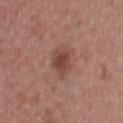Impression:
The lesion was photographed on a routine skin check and not biopsied; there is no pathology result.
Context:
This is a white-light tile. Longest diameter approximately 3.5 mm. Cropped from a total-body skin-imaging series; the visible field is about 15 mm. A male patient, approximately 30 years of age. The total-body-photography lesion software estimated a border-irregularity index near 2/10 and radial color variation of about 1.